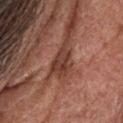Clinical impression:
No biopsy was performed on this lesion — it was imaged during a full skin examination and was not determined to be concerning.
Acquisition and patient details:
Cropped from a whole-body photographic skin survey; the tile spans about 15 mm. A male patient roughly 60 years of age. Automated tile analysis of the lesion measured a normalized lesion–skin contrast near 9. And it measured a classifier nevus-likeness of about 0/100 and lesion-presence confidence of about 60/100. Longest diameter approximately 3.5 mm. The lesion is located on the head or neck. This is a white-light tile.